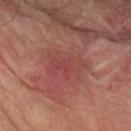  biopsy_status: not biopsied; imaged during a skin examination
  automated_metrics:
    color_variation_0_10: 3.5
    peripheral_color_asymmetry: 1.0
    nevus_likeness_0_100: 0
  lighting: cross-polarized
  site: left forearm
  lesion_size:
    long_diameter_mm_approx: 4.5
  patient:
    sex: male
    age_approx: 75
  image:
    source: total-body photography crop
    field_of_view_mm: 15